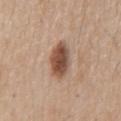Q: Is there a histopathology result?
A: total-body-photography surveillance lesion; no biopsy
Q: What is the imaging modality?
A: total-body-photography crop, ~15 mm field of view
Q: How large is the lesion?
A: ~4.5 mm (longest diameter)
Q: Patient demographics?
A: male, in their 60s
Q: Where on the body is the lesion?
A: the mid back